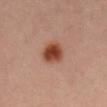Captured during whole-body skin photography for melanoma surveillance; the lesion was not biopsied. Approximately 3.5 mm at its widest. The total-body-photography lesion software estimated a footprint of about 8.5 mm², an eccentricity of roughly 0.45, and a symmetry-axis asymmetry near 0.15. The analysis additionally found an average lesion color of about L≈44 a*≈24 b*≈31 (CIELAB) and a lesion-to-skin contrast of about 11 (normalized; higher = more distinct). It also reported internal color variation of about 5 on a 0–10 scale and peripheral color asymmetry of about 1. A 15 mm close-up extracted from a 3D total-body photography capture. The lesion is located on the mid back. Captured under cross-polarized illumination. A male patient, aged 63–67.